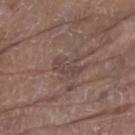Captured during whole-body skin photography for melanoma surveillance; the lesion was not biopsied. The total-body-photography lesion software estimated a lesion color around L≈42 a*≈15 b*≈20 in CIELAB and a normalized border contrast of about 5.5. A 15 mm close-up tile from a total-body photography series done for melanoma screening. Captured under white-light illumination. On the left lower leg. Longest diameter approximately 3.5 mm. The patient is a male aged 78 to 82.